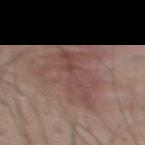This lesion was catalogued during total-body skin photography and was not selected for biopsy.
Longest diameter approximately 8 mm.
The lesion is on the mid back.
A male subject, aged around 50.
Imaged with white-light lighting.
Cropped from a whole-body photographic skin survey; the tile spans about 15 mm.
An algorithmic analysis of the crop reported an average lesion color of about L≈48 a*≈18 b*≈22 (CIELAB), about 7 CIELAB-L* units darker than the surrounding skin, and a normalized border contrast of about 5.5. It also reported a classifier nevus-likeness of about 0/100 and lesion-presence confidence of about 100/100.